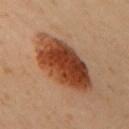Q: Was this lesion biopsied?
A: catalogued during a skin exam; not biopsied
Q: Who is the patient?
A: female, aged 58–62
Q: What kind of image is this?
A: ~15 mm tile from a whole-body skin photo
Q: Lesion location?
A: the upper back